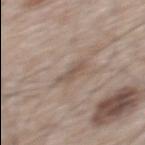illumination: white-light illumination
image: ~15 mm tile from a whole-body skin photo
lesion size: ~3 mm (longest diameter)
TBP lesion metrics: a within-lesion color-variation index near 0/10 and radial color variation of about 0
location: the upper back
subject: male, roughly 65 years of age